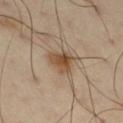<lesion>
  <biopsy_status>not biopsied; imaged during a skin examination</biopsy_status>
  <image>
    <source>total-body photography crop</source>
    <field_of_view_mm>15</field_of_view_mm>
  </image>
  <patient>
    <sex>male</sex>
    <age_approx>55</age_approx>
  </patient>
  <site>leg</site>
</lesion>